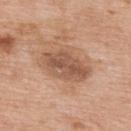biopsy status: imaged on a skin check; not biopsied | lighting: white-light | image source: ~15 mm crop, total-body skin-cancer survey | diameter: about 5.5 mm | body site: the upper back | subject: male, approximately 60 years of age.